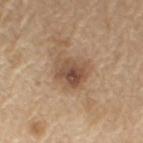The lesion was tiled from a total-body skin photograph and was not biopsied. The total-body-photography lesion software estimated an area of roughly 12 mm² and an eccentricity of roughly 0.4. The software also gave border irregularity of about 2.5 on a 0–10 scale, internal color variation of about 7.5 on a 0–10 scale, and peripheral color asymmetry of about 2.5. And it measured a classifier nevus-likeness of about 45/100 and a detector confidence of about 100 out of 100 that the crop contains a lesion. A region of skin cropped from a whole-body photographic capture, roughly 15 mm wide. This is a white-light tile. Located on the left upper arm. Measured at roughly 4 mm in maximum diameter. The patient is a male roughly 70 years of age.